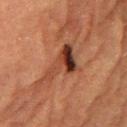Q: Was this lesion biopsied?
A: total-body-photography surveillance lesion; no biopsy
Q: How large is the lesion?
A: ≈5.5 mm
Q: What is the anatomic site?
A: the chest
Q: Patient demographics?
A: female, roughly 70 years of age
Q: How was the tile lit?
A: cross-polarized
Q: How was this image acquired?
A: ~15 mm tile from a whole-body skin photo